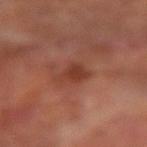follow-up=imaged on a skin check; not biopsied
image source=~15 mm crop, total-body skin-cancer survey
location=the right forearm
subject=male, roughly 70 years of age
automated lesion analysis=a lesion–skin lightness drop of about 8 and a normalized lesion–skin contrast near 6.5; a classifier nevus-likeness of about 5/100 and a detector confidence of about 100 out of 100 that the crop contains a lesion